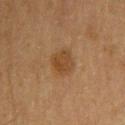Q: Is there a histopathology result?
A: total-body-photography surveillance lesion; no biopsy
Q: What did automated image analysis measure?
A: a footprint of about 7 mm² and a shape-asymmetry score of about 0.15 (0 = symmetric); about 7 CIELAB-L* units darker than the surrounding skin; lesion-presence confidence of about 100/100
Q: Lesion size?
A: ~3 mm (longest diameter)
Q: How was this image acquired?
A: 15 mm crop, total-body photography
Q: What are the patient's age and sex?
A: male, approximately 60 years of age